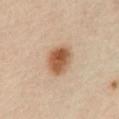Impression: Imaged during a routine full-body skin examination; the lesion was not biopsied and no histopathology is available. Context: The lesion is located on the abdomen. A male subject, in their mid- to late 60s. Cropped from a total-body skin-imaging series; the visible field is about 15 mm.